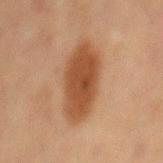{"biopsy_status": "not biopsied; imaged during a skin examination", "site": "front of the torso", "patient": {"sex": "male", "age_approx": 65}, "image": {"source": "total-body photography crop", "field_of_view_mm": 15}}